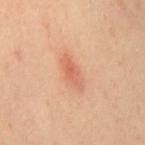Recorded during total-body skin imaging; not selected for excision or biopsy. From the mid back. About 4 mm across. A lesion tile, about 15 mm wide, cut from a 3D total-body photograph. A female subject, aged 38–42.